A lesion tile, about 15 mm wide, cut from a 3D total-body photograph; a male patient, aged approximately 75; on the left lower leg; Automated image analysis of the tile measured a footprint of about 0.5 mm² and an eccentricity of roughly 0.85. The software also gave a lesion color around L≈47 a*≈24 b*≈23 in CIELAB, about 6 CIELAB-L* units darker than the surrounding skin, and a lesion-to-skin contrast of about 5 (normalized; higher = more distinct). And it measured a classifier nevus-likeness of about 0/100; captured under white-light illumination:
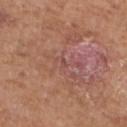Case summary:
- pathology: a nodular basal cell carcinoma